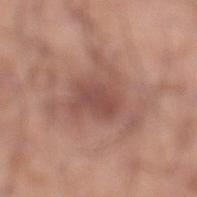Q: Is there a histopathology result?
A: no biopsy performed (imaged during a skin exam)
Q: Lesion location?
A: the leg
Q: Lesion size?
A: about 7.5 mm
Q: Who is the patient?
A: male, about 20 years old
Q: What kind of image is this?
A: 15 mm crop, total-body photography